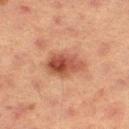Assessment:
Part of a total-body skin-imaging series; this lesion was reviewed on a skin check and was not flagged for biopsy.
Image and clinical context:
A female patient aged 53 to 57. A 15 mm close-up tile from a total-body photography series done for melanoma screening. Located on the left thigh. Automated tile analysis of the lesion measured a mean CIELAB color near L≈41 a*≈23 b*≈27, a lesion–skin lightness drop of about 11, and a lesion-to-skin contrast of about 9 (normalized; higher = more distinct). Imaged with cross-polarized lighting. Approximately 4 mm at its widest.